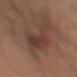{
  "biopsy_status": "not biopsied; imaged during a skin examination",
  "lesion_size": {
    "long_diameter_mm_approx": 7.0
  },
  "image": {
    "source": "total-body photography crop",
    "field_of_view_mm": 15
  },
  "patient": {
    "sex": "male",
    "age_approx": 55
  },
  "lighting": "white-light",
  "automated_metrics": {
    "cielab_L": 39,
    "cielab_a": 19,
    "cielab_b": 24,
    "vs_skin_darker_L": 7.0,
    "vs_skin_contrast_norm": 6.5,
    "border_irregularity_0_10": 6.0,
    "peripheral_color_asymmetry": 2.0
  },
  "site": "right upper arm"
}